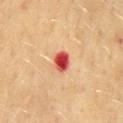follow-up: imaged on a skin check; not biopsied
TBP lesion metrics: a border-irregularity index near 2/10, a color-variation rating of about 5/10, and peripheral color asymmetry of about 1.5
lesion size: about 2.5 mm
location: the mid back
imaging modality: ~15 mm tile from a whole-body skin photo
subject: female, aged around 55
tile lighting: cross-polarized